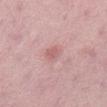Case summary:
- workup · catalogued during a skin exam; not biopsied
- body site · the abdomen
- patient · male, aged around 50
- acquisition · 15 mm crop, total-body photography
- lesion size · about 2.5 mm